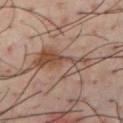Assessment: Captured during whole-body skin photography for melanoma surveillance; the lesion was not biopsied. Clinical summary: A 15 mm close-up tile from a total-body photography series done for melanoma screening. The lesion is located on the front of the torso. A male patient, aged around 40.